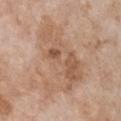TBP lesion metrics=an area of roughly 33 mm², a shape eccentricity near 0.85, and two-axis asymmetry of about 0.45; an automated nevus-likeness rating near 0 out of 100 and a detector confidence of about 100 out of 100 that the crop contains a lesion
location=the chest
subject=female, in their mid- to late 70s
imaging modality=~15 mm tile from a whole-body skin photo